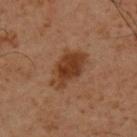No biopsy was performed on this lesion — it was imaged during a full skin examination and was not determined to be concerning.
The recorded lesion diameter is about 5.5 mm.
The total-body-photography lesion software estimated an average lesion color of about L≈37 a*≈21 b*≈31 (CIELAB), roughly 9 lightness units darker than nearby skin, and a normalized lesion–skin contrast near 8.5. The analysis additionally found border irregularity of about 3 on a 0–10 scale, a within-lesion color-variation index near 4/10, and radial color variation of about 1.5.
A 15 mm crop from a total-body photograph taken for skin-cancer surveillance.
The lesion is located on the back.
The patient is a male aged approximately 50.